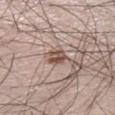workup=catalogued during a skin exam; not biopsied | body site=the left lower leg | automated metrics=a lesion color around L≈51 a*≈18 b*≈24 in CIELAB and about 13 CIELAB-L* units darker than the surrounding skin; internal color variation of about 6.5 on a 0–10 scale and peripheral color asymmetry of about 2.5; a nevus-likeness score of about 0/100 and a lesion-detection confidence of about 95/100 | imaging modality=~15 mm tile from a whole-body skin photo | size=≈3 mm | subject=male, in their mid-30s | illumination=white-light illumination.